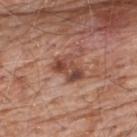Part of a total-body skin-imaging series; this lesion was reviewed on a skin check and was not flagged for biopsy. Captured under white-light illumination. The recorded lesion diameter is about 4 mm. A male patient aged 78 to 82. Cropped from a whole-body photographic skin survey; the tile spans about 15 mm. The lesion is located on the upper back.